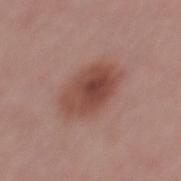Context: Imaged with white-light lighting. The lesion is located on the mid back. The patient is a male aged around 40. A lesion tile, about 15 mm wide, cut from a 3D total-body photograph. The lesion's longest dimension is about 5.5 mm. Diagnosis: Histopathological examination showed a dysplastic (Clark) nevus.